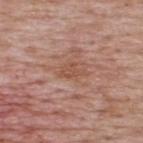follow-up: total-body-photography surveillance lesion; no biopsy
acquisition: 15 mm crop, total-body photography
subject: male, aged 63 to 67
lesion size: ~3 mm (longest diameter)
illumination: white-light
body site: the back
image-analysis metrics: a mean CIELAB color near L≈52 a*≈23 b*≈29, roughly 7 lightness units darker than nearby skin, and a lesion-to-skin contrast of about 6 (normalized; higher = more distinct)